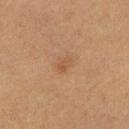follow-up: imaged on a skin check; not biopsied.